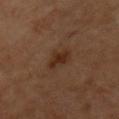No biopsy was performed on this lesion — it was imaged during a full skin examination and was not determined to be concerning. From the chest. Measured at roughly 3 mm in maximum diameter. A male subject, aged around 60. A close-up tile cropped from a whole-body skin photograph, about 15 mm across.